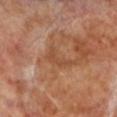biopsy status = catalogued during a skin exam; not biopsied
diameter = ~4.5 mm (longest diameter)
tile lighting = cross-polarized
location = the left lower leg
imaging modality = total-body-photography crop, ~15 mm field of view
patient = male, in their 70s